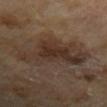Captured during whole-body skin photography for melanoma surveillance; the lesion was not biopsied.
This is a cross-polarized tile.
A male patient in their 70s.
The total-body-photography lesion software estimated a footprint of about 25 mm², an eccentricity of roughly 0.85, and a symmetry-axis asymmetry near 0.4.
A roughly 15 mm field-of-view crop from a total-body skin photograph.
Located on the left upper arm.
Longest diameter approximately 9 mm.